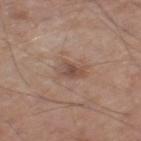biopsy status = no biopsy performed (imaged during a skin exam) | acquisition = ~15 mm crop, total-body skin-cancer survey | illumination = white-light | patient = male, in their mid- to late 60s | location = the leg | size = about 3 mm | TBP lesion metrics = an automated nevus-likeness rating near 5 out of 100 and lesion-presence confidence of about 100/100.